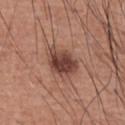follow-up: no biopsy performed (imaged during a skin exam)
image: 15 mm crop, total-body photography
patient: male, in their mid- to late 60s
location: the abdomen
tile lighting: white-light illumination
lesion size: about 4.5 mm